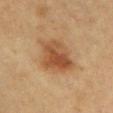The lesion was photographed on a routine skin check and not biopsied; there is no pathology result.
On the chest.
A female subject, aged 28–32.
Cropped from a whole-body photographic skin survey; the tile spans about 15 mm.